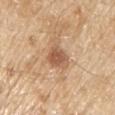Impression: No biopsy was performed on this lesion — it was imaged during a full skin examination and was not determined to be concerning. Image and clinical context: A male subject, about 70 years old. Automated image analysis of the tile measured an area of roughly 6.5 mm² and an outline eccentricity of about 0.55 (0 = round, 1 = elongated). The analysis additionally found a within-lesion color-variation index near 2.5/10 and a peripheral color-asymmetry measure near 1. The lesion is on the right upper arm. Cropped from a whole-body photographic skin survey; the tile spans about 15 mm. The lesion's longest dimension is about 3 mm. This is a white-light tile.